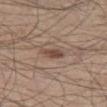{"biopsy_status": "not biopsied; imaged during a skin examination", "lighting": "white-light", "image": {"source": "total-body photography crop", "field_of_view_mm": 15}, "site": "left thigh", "patient": {"sex": "male", "age_approx": 45}}